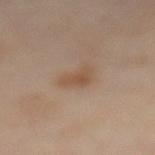workup: total-body-photography surveillance lesion; no biopsy | image: ~15 mm tile from a whole-body skin photo | lighting: cross-polarized illumination | automated metrics: an automated nevus-likeness rating near 25 out of 100 and a lesion-detection confidence of about 100/100 | site: the abdomen | diameter: ≈3 mm | patient: female, about 50 years old.